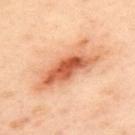biopsy status=total-body-photography surveillance lesion; no biopsy | anatomic site=the upper back | imaging modality=~15 mm crop, total-body skin-cancer survey | tile lighting=cross-polarized illumination | patient=female, roughly 40 years of age | size=≈8 mm.